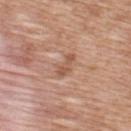Recorded during total-body skin imaging; not selected for excision or biopsy. On the upper back. A male subject aged 48–52. A 15 mm crop from a total-body photograph taken for skin-cancer surveillance. The lesion-visualizer software estimated a classifier nevus-likeness of about 0/100 and a detector confidence of about 100 out of 100 that the crop contains a lesion.